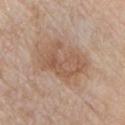follow-up: no biopsy performed (imaged during a skin exam)
patient: male, approximately 80 years of age
site: the front of the torso
image: 15 mm crop, total-body photography
size: ≈6 mm
automated lesion analysis: about 8 CIELAB-L* units darker than the surrounding skin and a normalized border contrast of about 6.5; a lesion-detection confidence of about 100/100